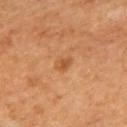Assessment: Recorded during total-body skin imaging; not selected for excision or biopsy. Image and clinical context: A male patient about 60 years old. The recorded lesion diameter is about 2.5 mm. A lesion tile, about 15 mm wide, cut from a 3D total-body photograph. This is a cross-polarized tile. On the back.